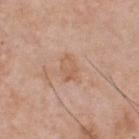Clinical impression:
No biopsy was performed on this lesion — it was imaged during a full skin examination and was not determined to be concerning.
Clinical summary:
This is a white-light tile. The lesion is on the chest. Automated image analysis of the tile measured a lesion–skin lightness drop of about 6. The software also gave a border-irregularity index near 3/10, internal color variation of about 3 on a 0–10 scale, and peripheral color asymmetry of about 1. It also reported lesion-presence confidence of about 100/100. The subject is a male aged around 50. The lesion's longest dimension is about 3 mm. Cropped from a whole-body photographic skin survey; the tile spans about 15 mm.